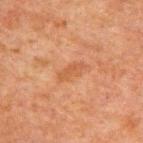The lesion was photographed on a routine skin check and not biopsied; there is no pathology result. A male subject aged 58–62. Located on the back. The lesion's longest dimension is about 3 mm. A 15 mm close-up extracted from a 3D total-body photography capture.